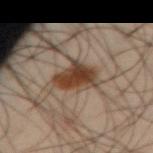Case summary:
* notes · imaged on a skin check; not biopsied
* subject · male, aged 43–47
* illumination · cross-polarized illumination
* lesion size · ~4.5 mm (longest diameter)
* anatomic site · the abdomen
* imaging modality · total-body-photography crop, ~15 mm field of view